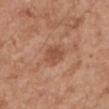patient: male, aged approximately 55; anatomic site: the arm; image source: total-body-photography crop, ~15 mm field of view; lighting: white-light illumination.